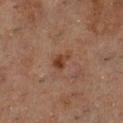  biopsy_status: not biopsied; imaged during a skin examination
  patient:
    sex: male
    age_approx: 50
  site: chest
  image:
    source: total-body photography crop
    field_of_view_mm: 15
  lesion_size:
    long_diameter_mm_approx: 3.0
  lighting: cross-polarized